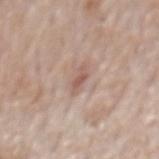{
  "biopsy_status": "not biopsied; imaged during a skin examination",
  "lesion_size": {
    "long_diameter_mm_approx": 3.0
  },
  "site": "back",
  "image": {
    "source": "total-body photography crop",
    "field_of_view_mm": 15
  },
  "patient": {
    "sex": "male",
    "age_approx": 70
  },
  "lighting": "white-light"
}